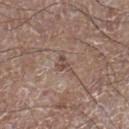  biopsy_status: not biopsied; imaged during a skin examination
  patient:
    sex: male
    age_approx: 65
  lesion_size:
    long_diameter_mm_approx: 3.0
  lighting: white-light
  site: right lower leg
  image:
    source: total-body photography crop
    field_of_view_mm: 15